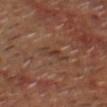The lesion was photographed on a routine skin check and not biopsied; there is no pathology result. Automated tile analysis of the lesion measured a lesion area of about 3.5 mm² and a shape-asymmetry score of about 0.5 (0 = symmetric). The software also gave a border-irregularity index near 5/10, internal color variation of about 1.5 on a 0–10 scale, and peripheral color asymmetry of about 0.5. And it measured a nevus-likeness score of about 0/100 and lesion-presence confidence of about 95/100. This image is a 15 mm lesion crop taken from a total-body photograph. The lesion is on the head or neck. A male subject aged approximately 60.